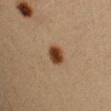biopsy status = total-body-photography surveillance lesion; no biopsy | body site = the left upper arm | patient = female, aged 28 to 32 | size = ≈3 mm | acquisition = ~15 mm crop, total-body skin-cancer survey | lighting = cross-polarized.